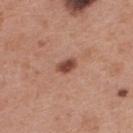Notes:
– follow-up · catalogued during a skin exam; not biopsied
– subject · female, roughly 40 years of age
– anatomic site · the upper back
– imaging modality · 15 mm crop, total-body photography